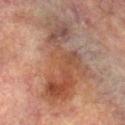The lesion-visualizer software estimated a shape-asymmetry score of about 0.55 (0 = symmetric). And it measured a mean CIELAB color near L≈40 a*≈18 b*≈25, roughly 8 lightness units darker than nearby skin, and a normalized border contrast of about 7. A male patient in their mid- to late 70s. Longest diameter approximately 10.5 mm. A 15 mm close-up tile from a total-body photography series done for melanoma screening. This is a cross-polarized tile. The lesion is located on the leg.